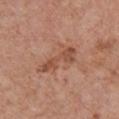Impression: This lesion was catalogued during total-body skin photography and was not selected for biopsy. Context: About 5.5 mm across. A region of skin cropped from a whole-body photographic capture, roughly 15 mm wide. A male subject, aged 78 to 82. Located on the chest. Captured under white-light illumination.